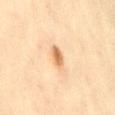Q: Was a biopsy performed?
A: imaged on a skin check; not biopsied
Q: Lesion size?
A: ≈2.5 mm
Q: How was the tile lit?
A: cross-polarized
Q: What are the patient's age and sex?
A: female, aged 43 to 47
Q: What did automated image analysis measure?
A: a footprint of about 4 mm², an outline eccentricity of about 0.75 (0 = round, 1 = elongated), and a shape-asymmetry score of about 0.2 (0 = symmetric); a border-irregularity index near 2/10 and internal color variation of about 3 on a 0–10 scale; lesion-presence confidence of about 100/100
Q: How was this image acquired?
A: ~15 mm tile from a whole-body skin photo
Q: What is the anatomic site?
A: the lower back